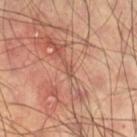  lesion_size:
    long_diameter_mm_approx: 2.5
  site: right thigh
  patient:
    sex: male
    age_approx: 60
  automated_metrics:
    area_mm2_approx: 1.5
    eccentricity: 0.9
    shape_asymmetry: 0.45
    border_irregularity_0_10: 4.5
    peripheral_color_asymmetry: 0.0
  image:
    source: total-body photography crop
    field_of_view_mm: 15